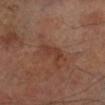| key | value |
|---|---|
| workup | imaged on a skin check; not biopsied |
| patient | male, approximately 70 years of age |
| acquisition | ~15 mm tile from a whole-body skin photo |
| TBP lesion metrics | a mean CIELAB color near L≈37 a*≈21 b*≈27 and a lesion-to-skin contrast of about 5.5 (normalized; higher = more distinct) |
| location | the right lower leg |
| lighting | cross-polarized illumination |
| lesion size | ~4 mm (longest diameter) |